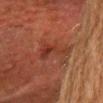* TBP lesion metrics: a mean CIELAB color near L≈29 a*≈23 b*≈26, about 6 CIELAB-L* units darker than the surrounding skin, and a normalized lesion–skin contrast near 7; a border-irregularity index near 6.5/10, internal color variation of about 2 on a 0–10 scale, and a peripheral color-asymmetry measure near 0.5; a classifier nevus-likeness of about 0/100
* illumination: cross-polarized
* location: the head or neck
* patient: male, in their mid-60s
* size: ≈4.5 mm
* acquisition: ~15 mm tile from a whole-body skin photo